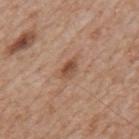Impression: This lesion was catalogued during total-body skin photography and was not selected for biopsy. Acquisition and patient details: A male subject, aged approximately 65. On the mid back. Cropped from a total-body skin-imaging series; the visible field is about 15 mm. Automated image analysis of the tile measured an area of roughly 3.5 mm², an outline eccentricity of about 0.8 (0 = round, 1 = elongated), and two-axis asymmetry of about 0.3. It also reported a lesion color around L≈50 a*≈21 b*≈30 in CIELAB. The software also gave a border-irregularity index near 2.5/10, a color-variation rating of about 4/10, and radial color variation of about 1.5. The analysis additionally found an automated nevus-likeness rating near 50 out of 100. Imaged with white-light lighting. The recorded lesion diameter is about 2.5 mm.